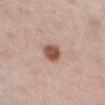| feature | finding |
|---|---|
| notes | no biopsy performed (imaged during a skin exam) |
| diameter | ~3 mm (longest diameter) |
| anatomic site | the right lower leg |
| image | total-body-photography crop, ~15 mm field of view |
| tile lighting | white-light |
| patient | female, aged 43 to 47 |